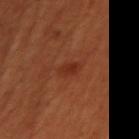Impression:
Part of a total-body skin-imaging series; this lesion was reviewed on a skin check and was not flagged for biopsy.
Clinical summary:
A female patient about 50 years old. A region of skin cropped from a whole-body photographic capture, roughly 15 mm wide.